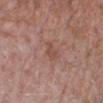Q: Is there a histopathology result?
A: total-body-photography surveillance lesion; no biopsy
Q: Lesion size?
A: ~2.5 mm (longest diameter)
Q: What is the anatomic site?
A: the right lower leg
Q: What kind of image is this?
A: 15 mm crop, total-body photography
Q: What lighting was used for the tile?
A: white-light illumination
Q: Patient demographics?
A: male, in their mid-80s or older